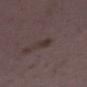The lesion was photographed on a routine skin check and not biopsied; there is no pathology result.
The lesion is on the right lower leg.
A close-up tile cropped from a whole-body skin photograph, about 15 mm across.
A female patient about 35 years old.
This is a white-light tile.
Approximately 2.5 mm at its widest.